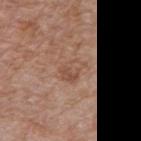Assessment:
The lesion was photographed on a routine skin check and not biopsied; there is no pathology result.
Image and clinical context:
The lesion is on the right upper arm. This is a white-light tile. A male patient about 70 years old. A 15 mm crop from a total-body photograph taken for skin-cancer surveillance. Longest diameter approximately 3.5 mm. The lesion-visualizer software estimated a mean CIELAB color near L≈52 a*≈20 b*≈28 and a normalized lesion–skin contrast near 5. It also reported a border-irregularity rating of about 3/10, a color-variation rating of about 5/10, and radial color variation of about 2. It also reported a classifier nevus-likeness of about 0/100.